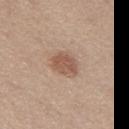Assessment:
Part of a total-body skin-imaging series; this lesion was reviewed on a skin check and was not flagged for biopsy.
Clinical summary:
The recorded lesion diameter is about 3.5 mm. The total-body-photography lesion software estimated an area of roughly 7.5 mm², an eccentricity of roughly 0.6, and two-axis asymmetry of about 0.15. The analysis additionally found a lesion-detection confidence of about 100/100. The tile uses white-light illumination. A roughly 15 mm field-of-view crop from a total-body skin photograph. On the left thigh. A female patient in their 40s.